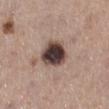Captured during whole-body skin photography for melanoma surveillance; the lesion was not biopsied.
The lesion-visualizer software estimated a footprint of about 12 mm², a shape eccentricity near 0.6, and two-axis asymmetry of about 0.15.
The lesion is on the right lower leg.
The tile uses white-light illumination.
A female subject, approximately 65 years of age.
A close-up tile cropped from a whole-body skin photograph, about 15 mm across.
Approximately 4.5 mm at its widest.